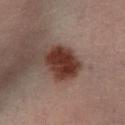- follow-up · total-body-photography surveillance lesion; no biopsy
- automated lesion analysis · a lesion color around L≈33 a*≈21 b*≈24 in CIELAB and a lesion–skin lightness drop of about 15; a border-irregularity index near 2/10 and radial color variation of about 1.5
- acquisition · 15 mm crop, total-body photography
- tile lighting · cross-polarized
- location · the left lower leg
- subject · male, aged 38–42